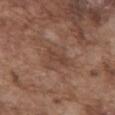Notes:
• biopsy status: total-body-photography surveillance lesion; no biopsy
• image-analysis metrics: a lesion area of about 2.5 mm², a shape eccentricity near 0.9, and a symmetry-axis asymmetry near 0.25; a mean CIELAB color near L≈41 a*≈19 b*≈26 and about 6 CIELAB-L* units darker than the surrounding skin; an automated nevus-likeness rating near 0 out of 100 and lesion-presence confidence of about 100/100
• diameter: ≈2.5 mm
• subject: male, approximately 75 years of age
• tile lighting: white-light illumination
• anatomic site: the abdomen
• imaging modality: ~15 mm crop, total-body skin-cancer survey